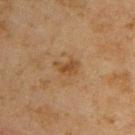workup: imaged on a skin check; not biopsied
patient: male, aged around 45
image source: total-body-photography crop, ~15 mm field of view
body site: the upper back
lesion diameter: ~3 mm (longest diameter)
image-analysis metrics: a lesion area of about 3.5 mm², an outline eccentricity of about 0.8 (0 = round, 1 = elongated), and two-axis asymmetry of about 0.4; a classifier nevus-likeness of about 5/100
tile lighting: cross-polarized